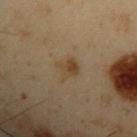Recorded during total-body skin imaging; not selected for excision or biopsy. This is a cross-polarized tile. An algorithmic analysis of the crop reported an eccentricity of roughly 0.65 and two-axis asymmetry of about 0.3. The software also gave a nevus-likeness score of about 50/100 and lesion-presence confidence of about 100/100. From the arm. A male patient about 55 years old. Cropped from a whole-body photographic skin survey; the tile spans about 15 mm. The recorded lesion diameter is about 3 mm.